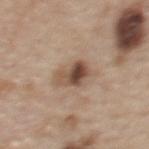<tbp_lesion>
<biopsy_status>not biopsied; imaged during a skin examination</biopsy_status>
<site>upper back</site>
<image>
  <source>total-body photography crop</source>
  <field_of_view_mm>15</field_of_view_mm>
</image>
<lighting>white-light</lighting>
<lesion_size>
  <long_diameter_mm_approx>4.0</long_diameter_mm_approx>
</lesion_size>
<patient>
  <sex>female</sex>
  <age_approx>50</age_approx>
</patient>
</tbp_lesion>